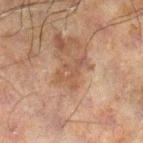This lesion was catalogued during total-body skin photography and was not selected for biopsy. This image is a 15 mm lesion crop taken from a total-body photograph. The lesion is on the left leg. The subject is a male aged around 60. Automated image analysis of the tile measured a lesion area of about 6.5 mm², a shape eccentricity near 0.8, and a symmetry-axis asymmetry near 0.6. Approximately 4 mm at its widest.